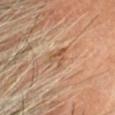This lesion was catalogued during total-body skin photography and was not selected for biopsy.
A roughly 15 mm field-of-view crop from a total-body skin photograph.
This is a cross-polarized tile.
The lesion-visualizer software estimated roughly 8 lightness units darker than nearby skin and a lesion-to-skin contrast of about 6 (normalized; higher = more distinct). And it measured a color-variation rating of about 4/10 and radial color variation of about 1.5. It also reported a nevus-likeness score of about 0/100 and a detector confidence of about 95 out of 100 that the crop contains a lesion.
A male subject, aged around 70.
The lesion is located on the head or neck.